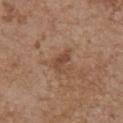A 15 mm crop from a total-body photograph taken for skin-cancer surveillance. Captured under white-light illumination. A female patient in their mid-60s. The lesion is located on the chest. The recorded lesion diameter is about 3 mm. The total-body-photography lesion software estimated lesion-presence confidence of about 100/100.